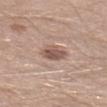Notes:
• follow-up — no biopsy performed (imaged during a skin exam)